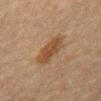Part of a total-body skin-imaging series; this lesion was reviewed on a skin check and was not flagged for biopsy. A 15 mm crop from a total-body photograph taken for skin-cancer surveillance. A male patient aged 58–62. The total-body-photography lesion software estimated a lesion area of about 9.5 mm², a shape eccentricity near 0.8, and two-axis asymmetry of about 0.15. And it measured about 7 CIELAB-L* units darker than the surrounding skin and a normalized lesion–skin contrast near 7.5. The software also gave border irregularity of about 2 on a 0–10 scale. The software also gave a classifier nevus-likeness of about 65/100 and a detector confidence of about 100 out of 100 that the crop contains a lesion. Measured at roughly 4.5 mm in maximum diameter. On the mid back. This is a cross-polarized tile.